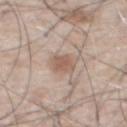Clinical summary: The lesion is on the front of the torso. Captured under white-light illumination. A close-up tile cropped from a whole-body skin photograph, about 15 mm across. A male patient, approximately 80 years of age. An algorithmic analysis of the crop reported a footprint of about 5.5 mm². The analysis additionally found about 9 CIELAB-L* units darker than the surrounding skin and a lesion-to-skin contrast of about 6.5 (normalized; higher = more distinct).